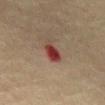Background: This image is a 15 mm lesion crop taken from a total-body photograph. Captured under cross-polarized illumination. Measured at roughly 3 mm in maximum diameter. The patient is a male aged 73 to 77. From the abdomen.